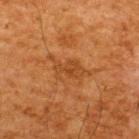Captured during whole-body skin photography for melanoma surveillance; the lesion was not biopsied.
A close-up tile cropped from a whole-body skin photograph, about 15 mm across.
From the back.
A male patient, about 65 years old.